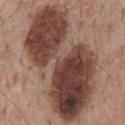Part of a total-body skin-imaging series; this lesion was reviewed on a skin check and was not flagged for biopsy. The subject is a male approximately 55 years of age. Located on the back. Automated tile analysis of the lesion measured border irregularity of about 6.5 on a 0–10 scale, a within-lesion color-variation index near 9/10, and a peripheral color-asymmetry measure near 3.5. The analysis additionally found a nevus-likeness score of about 0/100 and a detector confidence of about 100 out of 100 that the crop contains a lesion. Cropped from a total-body skin-imaging series; the visible field is about 15 mm.